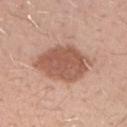Recorded during total-body skin imaging; not selected for excision or biopsy. Cropped from a whole-body photographic skin survey; the tile spans about 15 mm. A male patient, aged 28 to 32. The lesion is located on the right forearm.